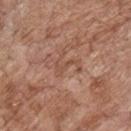follow-up = imaged on a skin check; not biopsied
anatomic site = the front of the torso
automated lesion analysis = an average lesion color of about L≈50 a*≈22 b*≈29 (CIELAB) and a normalized lesion–skin contrast near 5; a nevus-likeness score of about 0/100 and a detector confidence of about 95 out of 100 that the crop contains a lesion
lighting = white-light illumination
imaging modality = ~15 mm tile from a whole-body skin photo
subject = male, approximately 70 years of age